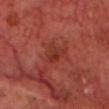Case summary:
* notes: catalogued during a skin exam; not biopsied
* automated metrics: roughly 6 lightness units darker than nearby skin and a lesion-to-skin contrast of about 6 (normalized; higher = more distinct); internal color variation of about 4 on a 0–10 scale; a nevus-likeness score of about 0/100 and a detector confidence of about 100 out of 100 that the crop contains a lesion
* lesion size: about 3.5 mm
* anatomic site: the head or neck
* subject: male, aged 68–72
* tile lighting: cross-polarized illumination
* acquisition: ~15 mm crop, total-body skin-cancer survey